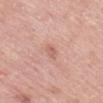{"biopsy_status": "not biopsied; imaged during a skin examination", "image": {"source": "total-body photography crop", "field_of_view_mm": 15}, "patient": {"sex": "male", "age_approx": 55}, "site": "mid back", "lighting": "white-light", "lesion_size": {"long_diameter_mm_approx": 2.5}}